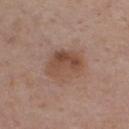workup = total-body-photography surveillance lesion; no biopsy | subject = male, aged 68 to 72 | size = about 4.5 mm | lighting = white-light | acquisition = total-body-photography crop, ~15 mm field of view | anatomic site = the chest | automated lesion analysis = an area of roughly 13 mm² and two-axis asymmetry of about 0.15; an average lesion color of about L≈48 a*≈19 b*≈28 (CIELAB), roughly 9 lightness units darker than nearby skin, and a normalized border contrast of about 7; a border-irregularity rating of about 2/10, internal color variation of about 6.5 on a 0–10 scale, and peripheral color asymmetry of about 2.5; a nevus-likeness score of about 20/100 and lesion-presence confidence of about 100/100.